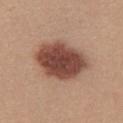Impression: This lesion was catalogued during total-body skin photography and was not selected for biopsy. Background: From the front of the torso. A female subject, in their mid-40s. This is a white-light tile. Automated tile analysis of the lesion measured an automated nevus-likeness rating near 100 out of 100 and a lesion-detection confidence of about 100/100. A 15 mm close-up tile from a total-body photography series done for melanoma screening.